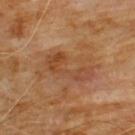Recorded during total-body skin imaging; not selected for excision or biopsy. Automated tile analysis of the lesion measured an area of roughly 9 mm², an eccentricity of roughly 0.95, and a symmetry-axis asymmetry near 0.55. The analysis additionally found a classifier nevus-likeness of about 0/100 and a lesion-detection confidence of about 100/100. This is a cross-polarized tile. The lesion is on the chest. A 15 mm close-up tile from a total-body photography series done for melanoma screening. The lesion's longest dimension is about 5.5 mm. A male subject in their 60s.